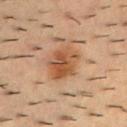About 4.5 mm across. Captured under cross-polarized illumination. From the back. A roughly 15 mm field-of-view crop from a total-body skin photograph. An algorithmic analysis of the crop reported a shape eccentricity near 0.6. It also reported a mean CIELAB color near L≈45 a*≈20 b*≈31, about 10 CIELAB-L* units darker than the surrounding skin, and a normalized lesion–skin contrast near 8. And it measured a border-irregularity rating of about 2/10, a color-variation rating of about 5.5/10, and radial color variation of about 2. The analysis additionally found a classifier nevus-likeness of about 95/100 and a lesion-detection confidence of about 100/100. A male subject roughly 55 years of age.